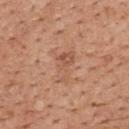Clinical impression: Part of a total-body skin-imaging series; this lesion was reviewed on a skin check and was not flagged for biopsy. Image and clinical context: On the back. The lesion-visualizer software estimated an area of roughly 8 mm² and an outline eccentricity of about 0.8 (0 = round, 1 = elongated). The software also gave an average lesion color of about L≈56 a*≈22 b*≈32 (CIELAB), roughly 7 lightness units darker than nearby skin, and a lesion-to-skin contrast of about 4.5 (normalized; higher = more distinct). Longest diameter approximately 4.5 mm. A 15 mm crop from a total-body photograph taken for skin-cancer surveillance. The patient is a male in their 60s.